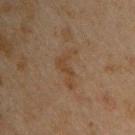No biopsy was performed on this lesion — it was imaged during a full skin examination and was not determined to be concerning. This image is a 15 mm lesion crop taken from a total-body photograph. The total-body-photography lesion software estimated a footprint of about 6.5 mm², an eccentricity of roughly 0.9, and a shape-asymmetry score of about 0.55 (0 = symmetric). It also reported roughly 5 lightness units darker than nearby skin and a normalized border contrast of about 5.5. From the chest. The lesion's longest dimension is about 4.5 mm. The patient is a male aged around 45.